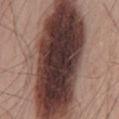The lesion was photographed on a routine skin check and not biopsied; there is no pathology result.
On the back.
A 15 mm close-up extracted from a 3D total-body photography capture.
A male patient approximately 55 years of age.